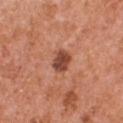<record>
<biopsy_status>not biopsied; imaged during a skin examination</biopsy_status>
<lighting>white-light</lighting>
<image>
  <source>total-body photography crop</source>
  <field_of_view_mm>15</field_of_view_mm>
</image>
<automated_metrics>
  <area_mm2_approx>5.5</area_mm2_approx>
  <eccentricity>0.65</eccentricity>
  <vs_skin_darker_L>14.0</vs_skin_darker_L>
  <vs_skin_contrast_norm>10.0</vs_skin_contrast_norm>
  <border_irregularity_0_10>2.0</border_irregularity_0_10>
  <peripheral_color_asymmetry>1.0</peripheral_color_asymmetry>
  <lesion_detection_confidence_0_100>100</lesion_detection_confidence_0_100>
</automated_metrics>
<patient>
  <sex>male</sex>
  <age_approx>55</age_approx>
</patient>
<site>chest</site>
</record>